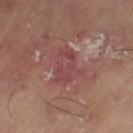Impression:
The lesion was photographed on a routine skin check and not biopsied; there is no pathology result.
Context:
On the left thigh. Captured under cross-polarized illumination. Approximately 4.5 mm at its widest. Cropped from a whole-body photographic skin survey; the tile spans about 15 mm.